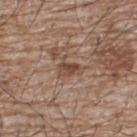site: back
patient:
  sex: male
  age_approx: 65
image:
  source: total-body photography crop
  field_of_view_mm: 15
automated_metrics:
  eccentricity: 0.75
  shape_asymmetry: 0.45
  border_irregularity_0_10: 5.0
  color_variation_0_10: 3.0
  peripheral_color_asymmetry: 1.0
lighting: white-light
lesion_size:
  long_diameter_mm_approx: 3.0